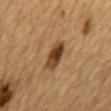- lesion size · ~4 mm (longest diameter)
- anatomic site · the mid back
- image source · total-body-photography crop, ~15 mm field of view
- subject · male, about 85 years old or older
- illumination · cross-polarized